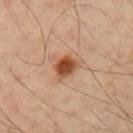workup = catalogued during a skin exam; not biopsied
patient = male, aged 48–52
image = ~15 mm tile from a whole-body skin photo
location = the left upper arm
illumination = cross-polarized illumination
diameter = ~3 mm (longest diameter)